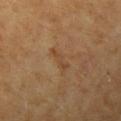{
  "biopsy_status": "not biopsied; imaged during a skin examination",
  "image": {
    "source": "total-body photography crop",
    "field_of_view_mm": 15
  },
  "lesion_size": {
    "long_diameter_mm_approx": 3.5
  },
  "lighting": "cross-polarized",
  "site": "arm",
  "patient": {
    "sex": "female",
    "age_approx": 60
  }
}